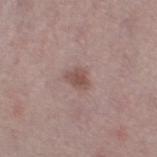No biopsy was performed on this lesion — it was imaged during a full skin examination and was not determined to be concerning. Longest diameter approximately 2.5 mm. A close-up tile cropped from a whole-body skin photograph, about 15 mm across. The patient is a female in their mid- to late 40s. The tile uses white-light illumination. On the leg. An algorithmic analysis of the crop reported a footprint of about 4 mm², an outline eccentricity of about 0.7 (0 = round, 1 = elongated), and a symmetry-axis asymmetry near 0.3. It also reported an average lesion color of about L≈50 a*≈19 b*≈22 (CIELAB), about 10 CIELAB-L* units darker than the surrounding skin, and a normalized lesion–skin contrast near 7.5. And it measured a detector confidence of about 100 out of 100 that the crop contains a lesion.